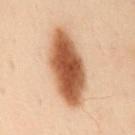Recorded during total-body skin imaging; not selected for excision or biopsy. From the mid back. This image is a 15 mm lesion crop taken from a total-body photograph. The patient is a female in their 60s. Imaged with cross-polarized lighting. Longest diameter approximately 9 mm.